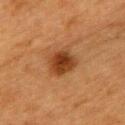biopsy status=total-body-photography surveillance lesion; no biopsy
subject=female, aged approximately 55
size=about 4 mm
image=15 mm crop, total-body photography
automated lesion analysis=a lesion color around L≈34 a*≈21 b*≈32 in CIELAB, about 11 CIELAB-L* units darker than the surrounding skin, and a normalized border contrast of about 9.5; a border-irregularity rating of about 2/10 and a color-variation rating of about 4.5/10; a nevus-likeness score of about 95/100 and a detector confidence of about 100 out of 100 that the crop contains a lesion
anatomic site=the upper back